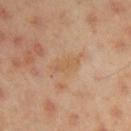The lesion was photographed on a routine skin check and not biopsied; there is no pathology result.
The lesion is located on the right upper arm.
A male subject, approximately 45 years of age.
A close-up tile cropped from a whole-body skin photograph, about 15 mm across.
Imaged with cross-polarized lighting.